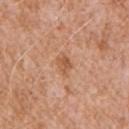Part of a total-body skin-imaging series; this lesion was reviewed on a skin check and was not flagged for biopsy. The patient is a male about 60 years old. Located on the right upper arm. An algorithmic analysis of the crop reported roughly 9 lightness units darker than nearby skin and a normalized lesion–skin contrast near 6.5. It also reported a border-irregularity rating of about 2.5/10, internal color variation of about 2 on a 0–10 scale, and a peripheral color-asymmetry measure near 1. This is a white-light tile. A lesion tile, about 15 mm wide, cut from a 3D total-body photograph.